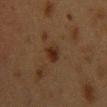| feature | finding |
|---|---|
| biopsy status | catalogued during a skin exam; not biopsied |
| tile lighting | cross-polarized illumination |
| patient | female, aged approximately 40 |
| size | about 2.5 mm |
| imaging modality | 15 mm crop, total-body photography |
| body site | the chest |